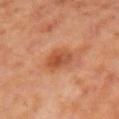Notes:
• biopsy status — total-body-photography surveillance lesion; no biopsy
• patient — female, about 50 years old
• lesion diameter — ≈3 mm
• image source — ~15 mm crop, total-body skin-cancer survey
• image-analysis metrics — a mean CIELAB color near L≈50 a*≈28 b*≈37, roughly 9 lightness units darker than nearby skin, and a normalized border contrast of about 7; a classifier nevus-likeness of about 70/100
• location — the arm
• illumination — cross-polarized